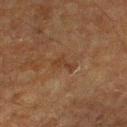{"biopsy_status": "not biopsied; imaged during a skin examination", "image": {"source": "total-body photography crop", "field_of_view_mm": 15}, "automated_metrics": {"cielab_L": 31, "cielab_a": 16, "cielab_b": 26, "vs_skin_darker_L": 5.0, "vs_skin_contrast_norm": 5.5, "border_irregularity_0_10": 6.0, "color_variation_0_10": 0.0, "peripheral_color_asymmetry": 0.0, "nevus_likeness_0_100": 0, "lesion_detection_confidence_0_100": 100}, "lesion_size": {"long_diameter_mm_approx": 3.0}, "site": "arm", "patient": {"sex": "male", "age_approx": 75}}